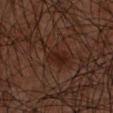biopsy_status: not biopsied; imaged during a skin examination
image:
  source: total-body photography crop
  field_of_view_mm: 15
lesion_size:
  long_diameter_mm_approx: 4.0
site: arm
lighting: cross-polarized
patient:
  sex: male
  age_approx: 65